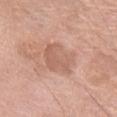follow-up: total-body-photography surveillance lesion; no biopsy | site: the left thigh | subject: female, in their mid-70s | image: total-body-photography crop, ~15 mm field of view.